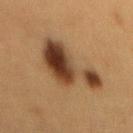Q: Is there a histopathology result?
A: total-body-photography surveillance lesion; no biopsy
Q: Lesion size?
A: ~7 mm (longest diameter)
Q: What is the imaging modality?
A: ~15 mm crop, total-body skin-cancer survey
Q: Where on the body is the lesion?
A: the back
Q: How was the tile lit?
A: cross-polarized illumination
Q: What are the patient's age and sex?
A: female, approximately 40 years of age